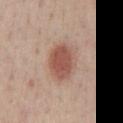patient = male, roughly 40 years of age
size = ≈4.5 mm
image source = 15 mm crop, total-body photography
site = the chest